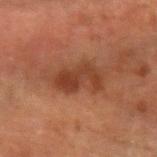<lesion>
  <biopsy_status>not biopsied; imaged during a skin examination</biopsy_status>
  <site>left forearm</site>
  <lighting>cross-polarized</lighting>
  <lesion_size>
    <long_diameter_mm_approx>5.0</long_diameter_mm_approx>
  </lesion_size>
  <image>
    <source>total-body photography crop</source>
    <field_of_view_mm>15</field_of_view_mm>
  </image>
  <patient>
    <sex>male</sex>
    <age_approx>60</age_approx>
  </patient>
</lesion>